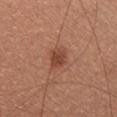The lesion-visualizer software estimated a lesion–skin lightness drop of about 10. The analysis additionally found border irregularity of about 2.5 on a 0–10 scale, a color-variation rating of about 2/10, and peripheral color asymmetry of about 0.5. A female subject in their mid- to late 20s. Located on the chest. The recorded lesion diameter is about 2.5 mm. Cropped from a total-body skin-imaging series; the visible field is about 15 mm.